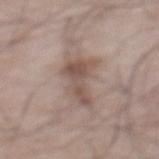Findings:
- follow-up · catalogued during a skin exam; not biopsied
- image · ~15 mm tile from a whole-body skin photo
- subject · male, aged around 65
- body site · the mid back
- lesion size · about 6 mm